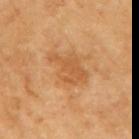follow-up — no biopsy performed (imaged during a skin exam); size — ≈4.5 mm; patient — female, about 70 years old; site — the right upper arm; image — ~15 mm tile from a whole-body skin photo; tile lighting — cross-polarized illumination.